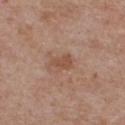Findings:
- workup — total-body-photography surveillance lesion; no biopsy
- illumination — white-light illumination
- acquisition — 15 mm crop, total-body photography
- location — the chest
- patient — male, aged 68–72
- diameter — ~3 mm (longest diameter)
- automated lesion analysis — an area of roughly 4 mm² and a shape eccentricity near 0.8; a border-irregularity index near 3/10 and radial color variation of about 0.5